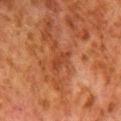Part of a total-body skin-imaging series; this lesion was reviewed on a skin check and was not flagged for biopsy. The lesion's longest dimension is about 3 mm. The total-body-photography lesion software estimated a lesion color around L≈34 a*≈24 b*≈31 in CIELAB and about 6 CIELAB-L* units darker than the surrounding skin. And it measured an automated nevus-likeness rating near 0 out of 100 and a detector confidence of about 100 out of 100 that the crop contains a lesion. On the right lower leg. Imaged with cross-polarized lighting. A close-up tile cropped from a whole-body skin photograph, about 15 mm across. A male subject, in their 80s.